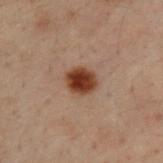Captured during whole-body skin photography for melanoma surveillance; the lesion was not biopsied. From the upper back. A roughly 15 mm field-of-view crop from a total-body skin photograph. A male patient aged 28–32.